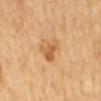Notes:
- follow-up — total-body-photography surveillance lesion; no biopsy
- TBP lesion metrics — border irregularity of about 4 on a 0–10 scale; an automated nevus-likeness rating near 10 out of 100 and a lesion-detection confidence of about 100/100
- lesion diameter — ≈3 mm
- body site — the mid back
- tile lighting — cross-polarized
- patient — male, about 65 years old
- imaging modality — ~15 mm tile from a whole-body skin photo